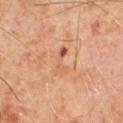This lesion was catalogued during total-body skin photography and was not selected for biopsy. Captured under cross-polarized illumination. A male patient, aged around 65. The total-body-photography lesion software estimated a footprint of about 4.5 mm², an eccentricity of roughly 0.95, and a symmetry-axis asymmetry near 0.45. The software also gave a border-irregularity index near 6/10, a color-variation rating of about 5/10, and peripheral color asymmetry of about 0. Measured at roughly 4 mm in maximum diameter. A 15 mm close-up extracted from a 3D total-body photography capture.